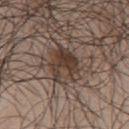<case>
<biopsy_status>not biopsied; imaged during a skin examination</biopsy_status>
<site>back</site>
<patient>
  <sex>male</sex>
  <age_approx>50</age_approx>
</patient>
<image>
  <source>total-body photography crop</source>
  <field_of_view_mm>15</field_of_view_mm>
</image>
</case>